A 15 mm crop from a total-body photograph taken for skin-cancer surveillance · from the right forearm · a female patient in their mid-20s — 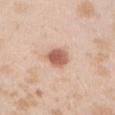Imaged with white-light lighting.
The total-body-photography lesion software estimated a footprint of about 6.5 mm². And it measured a classifier nevus-likeness of about 100/100 and a detector confidence of about 100 out of 100 that the crop contains a lesion.
Longest diameter approximately 3 mm.
Biopsy histopathology demonstrated a dysplastic (Clark) nevus.